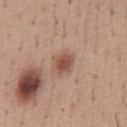Q: Is there a histopathology result?
A: total-body-photography surveillance lesion; no biopsy
Q: Automated lesion metrics?
A: a footprint of about 5 mm², an eccentricity of roughly 0.6, and a shape-asymmetry score of about 0.15 (0 = symmetric); a border-irregularity rating of about 1.5/10, internal color variation of about 3.5 on a 0–10 scale, and peripheral color asymmetry of about 1; lesion-presence confidence of about 100/100
Q: Who is the patient?
A: male, about 40 years old
Q: How large is the lesion?
A: ≈2.5 mm
Q: Where on the body is the lesion?
A: the abdomen
Q: How was the tile lit?
A: white-light
Q: What is the imaging modality?
A: ~15 mm crop, total-body skin-cancer survey